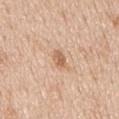Q: Was this lesion biopsied?
A: imaged on a skin check; not biopsied
Q: How was this image acquired?
A: total-body-photography crop, ~15 mm field of view
Q: What is the anatomic site?
A: the chest
Q: What are the patient's age and sex?
A: male, in their mid- to late 60s